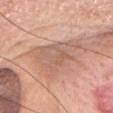| key | value |
|---|---|
| follow-up | imaged on a skin check; not biopsied |
| acquisition | ~15 mm tile from a whole-body skin photo |
| lighting | white-light illumination |
| patient | female, about 65 years old |
| anatomic site | the head or neck |
| diameter | ~7 mm (longest diameter) |